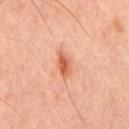{
  "biopsy_status": "not biopsied; imaged during a skin examination",
  "lesion_size": {
    "long_diameter_mm_approx": 3.0
  },
  "site": "back",
  "patient": {
    "sex": "male",
    "age_approx": 45
  },
  "lighting": "cross-polarized",
  "automated_metrics": {
    "area_mm2_approx": 4.5,
    "shape_asymmetry": 0.35,
    "cielab_L": 57,
    "cielab_a": 27,
    "cielab_b": 36,
    "border_irregularity_0_10": 3.5,
    "color_variation_0_10": 3.5,
    "peripheral_color_asymmetry": 1.0
  },
  "image": {
    "source": "total-body photography crop",
    "field_of_view_mm": 15
  }
}